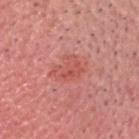notes — catalogued during a skin exam; not biopsied
image source — 15 mm crop, total-body photography
automated metrics — a lesion area of about 8.5 mm², an eccentricity of roughly 0.8, and a shape-asymmetry score of about 0.3 (0 = symmetric); an average lesion color of about L≈55 a*≈32 b*≈29 (CIELAB), a lesion–skin lightness drop of about 7, and a lesion-to-skin contrast of about 5.5 (normalized; higher = more distinct); border irregularity of about 4.5 on a 0–10 scale, internal color variation of about 4 on a 0–10 scale, and radial color variation of about 1.5; a nevus-likeness score of about 5/100 and a detector confidence of about 100 out of 100 that the crop contains a lesion
subject — male, about 60 years old
lighting — white-light
diameter — about 4.5 mm
location — the head or neck